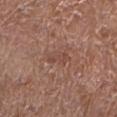| field | value |
|---|---|
| workup | catalogued during a skin exam; not biopsied |
| illumination | white-light |
| location | the left lower leg |
| size | about 3 mm |
| patient | female, about 80 years old |
| image source | total-body-photography crop, ~15 mm field of view |